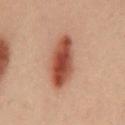Q: Was a biopsy performed?
A: catalogued during a skin exam; not biopsied
Q: How was this image acquired?
A: total-body-photography crop, ~15 mm field of view
Q: Patient demographics?
A: male, approximately 40 years of age
Q: Automated lesion metrics?
A: an average lesion color of about L≈39 a*≈22 b*≈26 (CIELAB) and roughly 13 lightness units darker than nearby skin; a nevus-likeness score of about 100/100 and a detector confidence of about 100 out of 100 that the crop contains a lesion
Q: Where on the body is the lesion?
A: the chest
Q: How large is the lesion?
A: ~6.5 mm (longest diameter)
Q: How was the tile lit?
A: cross-polarized